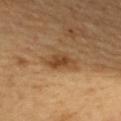biopsy_status: not biopsied; imaged during a skin examination
lesion_size:
  long_diameter_mm_approx: 4.5
lighting: cross-polarized
site: upper back
image:
  source: total-body photography crop
  field_of_view_mm: 15
patient:
  sex: female
  age_approx: 40